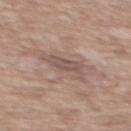Assessment: Imaged during a routine full-body skin examination; the lesion was not biopsied and no histopathology is available. Background: A 15 mm close-up tile from a total-body photography series done for melanoma screening. A male subject aged around 60. Located on the chest. Measured at roughly 4.5 mm in maximum diameter. This is a white-light tile.